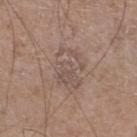Impression: Recorded during total-body skin imaging; not selected for excision or biopsy. Context: Captured under white-light illumination. On the leg. Automated tile analysis of the lesion measured a mean CIELAB color near L≈52 a*≈15 b*≈22, about 6 CIELAB-L* units darker than the surrounding skin, and a lesion-to-skin contrast of about 4.5 (normalized; higher = more distinct). The software also gave a border-irregularity rating of about 5/10, internal color variation of about 4.5 on a 0–10 scale, and radial color variation of about 1.5. The analysis additionally found a classifier nevus-likeness of about 0/100 and a detector confidence of about 100 out of 100 that the crop contains a lesion. Longest diameter approximately 5 mm. A 15 mm crop from a total-body photograph taken for skin-cancer surveillance. A male patient in their mid-60s.